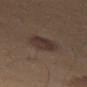Q: Was this lesion biopsied?
A: catalogued during a skin exam; not biopsied
Q: What is the imaging modality?
A: ~15 mm tile from a whole-body skin photo
Q: Lesion location?
A: the right thigh
Q: What lighting was used for the tile?
A: cross-polarized
Q: Automated lesion metrics?
A: a lesion area of about 9.5 mm², an outline eccentricity of about 0.7 (0 = round, 1 = elongated), and two-axis asymmetry of about 0.2; a lesion color around L≈32 a*≈14 b*≈19 in CIELAB and about 8 CIELAB-L* units darker than the surrounding skin; a border-irregularity index near 2/10 and a peripheral color-asymmetry measure near 1; a nevus-likeness score of about 80/100 and a lesion-detection confidence of about 100/100
Q: How large is the lesion?
A: ≈4 mm
Q: What are the patient's age and sex?
A: male, approximately 55 years of age